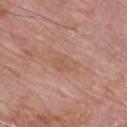notes: imaged on a skin check; not biopsied